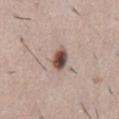The lesion was tiled from a total-body skin photograph and was not biopsied. Cropped from a whole-body photographic skin survey; the tile spans about 15 mm. The total-body-photography lesion software estimated a lesion color around L≈50 a*≈17 b*≈23 in CIELAB and a lesion-to-skin contrast of about 12 (normalized; higher = more distinct). The analysis additionally found a border-irregularity rating of about 2/10, a color-variation rating of about 7.5/10, and a peripheral color-asymmetry measure near 2. It also reported a classifier nevus-likeness of about 100/100. A male subject in their 50s. Imaged with white-light lighting. Located on the abdomen.